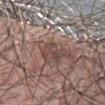Q: Was a biopsy performed?
A: no biopsy performed (imaged during a skin exam)
Q: What are the patient's age and sex?
A: male, in their mid- to late 70s
Q: Automated lesion metrics?
A: a lesion-detection confidence of about 95/100
Q: What is the imaging modality?
A: ~15 mm tile from a whole-body skin photo
Q: Where on the body is the lesion?
A: the left forearm
Q: Illumination type?
A: white-light